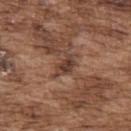Impression: Imaged during a routine full-body skin examination; the lesion was not biopsied and no histopathology is available. Context: Cropped from a total-body skin-imaging series; the visible field is about 15 mm. The lesion is on the upper back. The recorded lesion diameter is about 3 mm. A male patient aged around 75. The tile uses white-light illumination.